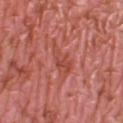workup = catalogued during a skin exam; not biopsied | image source = ~15 mm crop, total-body skin-cancer survey | patient = female, approximately 40 years of age | body site = the right lower leg | lighting = white-light | diameter = about 4 mm.